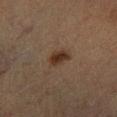Imaged during a routine full-body skin examination; the lesion was not biopsied and no histopathology is available.
The tile uses cross-polarized illumination.
A male patient, aged 83 or over.
This image is a 15 mm lesion crop taken from a total-body photograph.
On the right lower leg.
Measured at roughly 2.5 mm in maximum diameter.